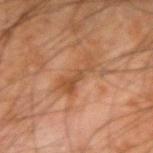The lesion was tiled from a total-body skin photograph and was not biopsied. A male subject aged 63–67. Imaged with cross-polarized lighting. The lesion is located on the right forearm. This image is a 15 mm lesion crop taken from a total-body photograph. About 4.5 mm across. Automated image analysis of the tile measured a mean CIELAB color near L≈43 a*≈20 b*≈32 and about 7 CIELAB-L* units darker than the surrounding skin. The software also gave a nevus-likeness score of about 0/100 and a detector confidence of about 100 out of 100 that the crop contains a lesion.